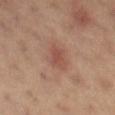Part of a total-body skin-imaging series; this lesion was reviewed on a skin check and was not flagged for biopsy.
On the left thigh.
A female subject, about 40 years old.
A 15 mm close-up extracted from a 3D total-body photography capture.